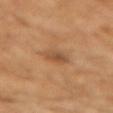{
  "biopsy_status": "not biopsied; imaged during a skin examination",
  "image": {
    "source": "total-body photography crop",
    "field_of_view_mm": 15
  },
  "patient": {
    "sex": "female",
    "age_approx": 70
  },
  "lesion_size": {
    "long_diameter_mm_approx": 3.0
  },
  "lighting": "cross-polarized",
  "site": "left forearm"
}